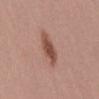Background: The patient is a female roughly 25 years of age. Cropped from a total-body skin-imaging series; the visible field is about 15 mm. From the back.